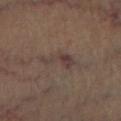This lesion was catalogued during total-body skin photography and was not selected for biopsy.
Imaged with cross-polarized lighting.
The recorded lesion diameter is about 4.5 mm.
A lesion tile, about 15 mm wide, cut from a 3D total-body photograph.
The subject is aged 63–67.
The lesion is located on the right lower leg.
The total-body-photography lesion software estimated a footprint of about 5.5 mm², a shape eccentricity near 0.9, and a shape-asymmetry score of about 0.65 (0 = symmetric).